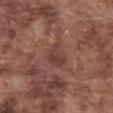| key | value |
|---|---|
| imaging modality | ~15 mm crop, total-body skin-cancer survey |
| tile lighting | white-light |
| patient | male, approximately 75 years of age |
| site | the front of the torso |
| lesion diameter | ≈3 mm |